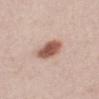Assessment:
Captured during whole-body skin photography for melanoma surveillance; the lesion was not biopsied.
Clinical summary:
The lesion is on the mid back. A male patient, approximately 40 years of age. Cropped from a whole-body photographic skin survey; the tile spans about 15 mm. Automated tile analysis of the lesion measured a detector confidence of about 100 out of 100 that the crop contains a lesion.